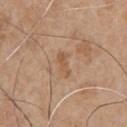No biopsy was performed on this lesion — it was imaged during a full skin examination and was not determined to be concerning. The subject is a male in their mid- to late 60s. Captured under white-light illumination. The lesion is located on the chest. This image is a 15 mm lesion crop taken from a total-body photograph.